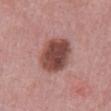Case summary:
– workup — catalogued during a skin exam; not biopsied
– lesion diameter — ~5 mm (longest diameter)
– body site — the abdomen
– subject — male, roughly 50 years of age
– illumination — white-light illumination
– image — ~15 mm tile from a whole-body skin photo